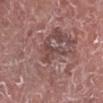{"biopsy_status": "not biopsied; imaged during a skin examination", "patient": {"sex": "male", "age_approx": 75}, "automated_metrics": {"cielab_L": 46, "cielab_a": 20, "cielab_b": 20, "vs_skin_darker_L": 7.0, "vs_skin_contrast_norm": 5.5, "border_irregularity_0_10": 8.5, "color_variation_0_10": 1.5}, "image": {"source": "total-body photography crop", "field_of_view_mm": 15}, "lesion_size": {"long_diameter_mm_approx": 4.0}, "site": "right lower leg"}